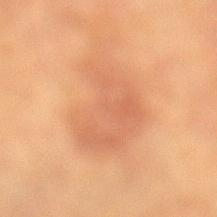lesion diameter: about 8.5 mm | site: the left lower leg | acquisition: ~15 mm tile from a whole-body skin photo | patient: male, aged 83 or older.